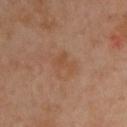Clinical impression:
No biopsy was performed on this lesion — it was imaged during a full skin examination and was not determined to be concerning.
Background:
Captured under cross-polarized illumination. A 15 mm close-up extracted from a 3D total-body photography capture. Measured at roughly 2.5 mm in maximum diameter. A male patient, aged 38 to 42. Located on the chest.